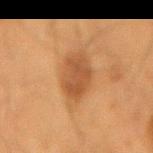Assessment: This lesion was catalogued during total-body skin photography and was not selected for biopsy. Image and clinical context: The tile uses cross-polarized illumination. A male patient aged 63–67. A close-up tile cropped from a whole-body skin photograph, about 15 mm across. Located on the lower back.